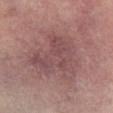Impression: Captured during whole-body skin photography for melanoma surveillance; the lesion was not biopsied. Clinical summary: Automated image analysis of the tile measured an eccentricity of roughly 0.6 and two-axis asymmetry of about 0.45. And it measured a lesion color around L≈45 a*≈20 b*≈17 in CIELAB, about 7 CIELAB-L* units darker than the surrounding skin, and a normalized border contrast of about 6. The software also gave an automated nevus-likeness rating near 0 out of 100 and a detector confidence of about 95 out of 100 that the crop contains a lesion. Measured at roughly 6.5 mm in maximum diameter. The subject is a male aged 63 to 67. The lesion is on the left lower leg. Imaged with cross-polarized lighting. A close-up tile cropped from a whole-body skin photograph, about 15 mm across.